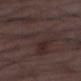Findings:
– workup · catalogued during a skin exam; not biopsied
– anatomic site · the left lower leg
– image source · 15 mm crop, total-body photography
– patient · male, aged 48 to 52